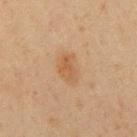illumination = cross-polarized illumination | size = ≈4.5 mm | anatomic site = the mid back | image = ~15 mm tile from a whole-body skin photo | subject = male, roughly 60 years of age.